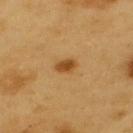  image:
    source: total-body photography crop
    field_of_view_mm: 15
  site: upper back
  automated_metrics:
    shape_asymmetry: 0.2
    nevus_likeness_0_100: 100
  patient:
    sex: male
    age_approx: 60
  lighting: cross-polarized